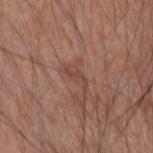Recorded during total-body skin imaging; not selected for excision or biopsy. A male patient, about 55 years old. Cropped from a whole-body photographic skin survey; the tile spans about 15 mm. About 3 mm across. Located on the left forearm. Captured under white-light illumination.